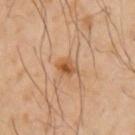biopsy status — no biopsy performed (imaged during a skin exam)
imaging modality — ~15 mm crop, total-body skin-cancer survey
location — the left upper arm
automated metrics — a border-irregularity index near 1.5/10, a color-variation rating of about 6/10, and radial color variation of about 2; a classifier nevus-likeness of about 90/100
diameter — about 2.5 mm
subject — male, aged around 50
lighting — cross-polarized illumination